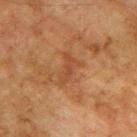The subject is a male roughly 75 years of age. A region of skin cropped from a whole-body photographic capture, roughly 15 mm wide. This is a cross-polarized tile. On the back. The total-body-photography lesion software estimated a border-irregularity index near 6/10, a color-variation rating of about 2/10, and a peripheral color-asymmetry measure near 0.5. And it measured a nevus-likeness score of about 0/100 and lesion-presence confidence of about 100/100.